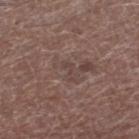Assessment: The lesion was photographed on a routine skin check and not biopsied; there is no pathology result. Image and clinical context: Automated image analysis of the tile measured a lesion area of about 2 mm² and a shape-asymmetry score of about 0.55 (0 = symmetric). It also reported a border-irregularity index near 7/10, a color-variation rating of about 0/10, and peripheral color asymmetry of about 0. And it measured an automated nevus-likeness rating near 0 out of 100. Captured under white-light illumination. On the right lower leg. The lesion's longest dimension is about 2.5 mm. A region of skin cropped from a whole-body photographic capture, roughly 15 mm wide. The patient is a male aged approximately 75.